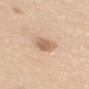| key | value |
|---|---|
| workup | no biopsy performed (imaged during a skin exam) |
| tile lighting | white-light illumination |
| site | the mid back |
| acquisition | ~15 mm tile from a whole-body skin photo |
| subject | female, aged around 65 |